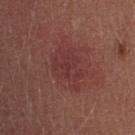| key | value |
|---|---|
| follow-up | total-body-photography surveillance lesion; no biopsy |
| subject | female, approximately 25 years of age |
| location | the upper back |
| lesion diameter | ~5.5 mm (longest diameter) |
| imaging modality | 15 mm crop, total-body photography |
| lighting | white-light |